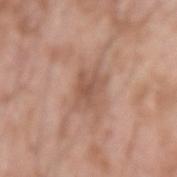follow-up = catalogued during a skin exam; not biopsied
imaging modality = 15 mm crop, total-body photography
image-analysis metrics = border irregularity of about 4 on a 0–10 scale and radial color variation of about 0.5; a classifier nevus-likeness of about 0/100 and a lesion-detection confidence of about 100/100
patient = male, aged around 60
illumination = white-light
lesion diameter = about 3.5 mm
anatomic site = the arm